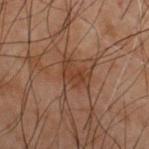• follow-up — total-body-photography surveillance lesion; no biopsy
• lesion diameter — about 3.5 mm
• TBP lesion metrics — a footprint of about 4.5 mm² and two-axis asymmetry of about 0.55; border irregularity of about 6 on a 0–10 scale, internal color variation of about 1 on a 0–10 scale, and radial color variation of about 0.5; a nevus-likeness score of about 0/100 and a detector confidence of about 100 out of 100 that the crop contains a lesion
• patient — male, approximately 70 years of age
• imaging modality — 15 mm crop, total-body photography
• site — the chest
• illumination — cross-polarized illumination